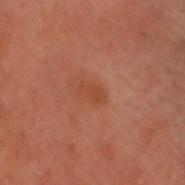workup: imaged on a skin check; not biopsied
body site: the head or neck
subject: male, approximately 50 years of age
imaging modality: ~15 mm crop, total-body skin-cancer survey
lesion size: about 2.5 mm